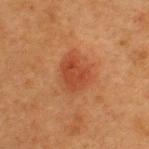Notes:
• notes: no biopsy performed (imaged during a skin exam)
• image: 15 mm crop, total-body photography
• anatomic site: the upper back
• tile lighting: cross-polarized illumination
• TBP lesion metrics: a mean CIELAB color near L≈40 a*≈27 b*≈34 and about 8 CIELAB-L* units darker than the surrounding skin; a border-irregularity index near 3/10, a color-variation rating of about 2.5/10, and radial color variation of about 1; an automated nevus-likeness rating near 95 out of 100 and a lesion-detection confidence of about 100/100
• patient: female, roughly 40 years of age